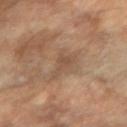This lesion was catalogued during total-body skin photography and was not selected for biopsy.
The lesion's longest dimension is about 3.5 mm.
A 15 mm close-up extracted from a 3D total-body photography capture.
Captured under cross-polarized illumination.
From the right forearm.
The total-body-photography lesion software estimated a footprint of about 5.5 mm², an eccentricity of roughly 0.7, and a shape-asymmetry score of about 0.5 (0 = symmetric). And it measured a mean CIELAB color near L≈51 a*≈17 b*≈30 and a normalized border contrast of about 5. And it measured a border-irregularity index near 5.5/10, a within-lesion color-variation index near 2/10, and a peripheral color-asymmetry measure near 0.5.
A female subject, aged around 70.